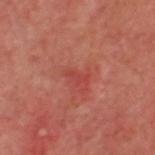workup = imaged on a skin check; not biopsied | subject = male, approximately 60 years of age | lesion diameter = ~2.5 mm (longest diameter) | illumination = cross-polarized illumination | location = the head or neck | acquisition = 15 mm crop, total-body photography.